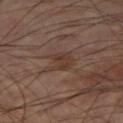Imaged during a routine full-body skin examination; the lesion was not biopsied and no histopathology is available. Longest diameter approximately 3.5 mm. Automated image analysis of the tile measured a lesion area of about 6.5 mm², a shape eccentricity near 0.7, and a symmetry-axis asymmetry near 0.4. The software also gave a lesion color around L≈35 a*≈16 b*≈24 in CIELAB. The analysis additionally found a within-lesion color-variation index near 2.5/10 and a peripheral color-asymmetry measure near 1. It also reported a nevus-likeness score of about 0/100 and lesion-presence confidence of about 100/100. A region of skin cropped from a whole-body photographic capture, roughly 15 mm wide. This is a cross-polarized tile. A male subject, in their 60s. From the right thigh.